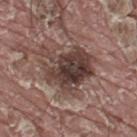Findings:
* notes: no biopsy performed (imaged during a skin exam)
* patient: male, aged 38–42
* diameter: ≈7 mm
* body site: the upper back
* tile lighting: white-light illumination
* acquisition: ~15 mm crop, total-body skin-cancer survey